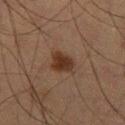Assessment: Recorded during total-body skin imaging; not selected for excision or biopsy. Background: The subject is a male aged approximately 65. Located on the left thigh. A close-up tile cropped from a whole-body skin photograph, about 15 mm across.